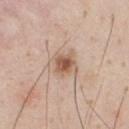The lesion's longest dimension is about 2.5 mm.
Automated image analysis of the tile measured a border-irregularity rating of about 2/10, a within-lesion color-variation index near 4/10, and a peripheral color-asymmetry measure near 1. The analysis additionally found a classifier nevus-likeness of about 90/100 and a lesion-detection confidence of about 100/100.
The tile uses white-light illumination.
A male patient, aged around 45.
Located on the front of the torso.
A 15 mm close-up extracted from a 3D total-body photography capture.